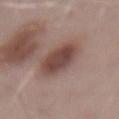Clinical impression: The lesion was tiled from a total-body skin photograph and was not biopsied. Context: Imaged with white-light lighting. A male subject, in their mid-50s. The lesion is on the mid back. The lesion-visualizer software estimated a border-irregularity index near 2/10 and peripheral color asymmetry of about 1.5. And it measured a classifier nevus-likeness of about 80/100 and a detector confidence of about 100 out of 100 that the crop contains a lesion. The recorded lesion diameter is about 6.5 mm. A close-up tile cropped from a whole-body skin photograph, about 15 mm across.